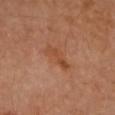  patient:
    sex: female
    age_approx: 65
  site: left forearm
  automated_metrics:
    cielab_L: 48
    cielab_a: 25
    cielab_b: 35
    vs_skin_darker_L: 7.0
    vs_skin_contrast_norm: 6.0
    nevus_likeness_0_100: 0
    lesion_detection_confidence_0_100: 100
  lesion_size:
    long_diameter_mm_approx: 4.0
  lighting: cross-polarized
  image:
    source: total-body photography crop
    field_of_view_mm: 15